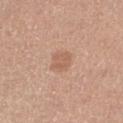The lesion was photographed on a routine skin check and not biopsied; there is no pathology result.
From the right thigh.
A roughly 15 mm field-of-view crop from a total-body skin photograph.
About 2.5 mm across.
Imaged with white-light lighting.
A female patient about 60 years old.